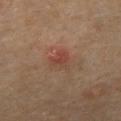Imaged during a routine full-body skin examination; the lesion was not biopsied and no histopathology is available.
A male patient, aged around 65.
A roughly 15 mm field-of-view crop from a total-body skin photograph.
The lesion is on the abdomen.